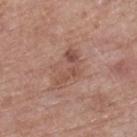Image and clinical context: A 15 mm crop from a total-body photograph taken for skin-cancer surveillance. A female patient, aged around 70. The lesion is on the right lower leg. Approximately 4.5 mm at its widest. Captured under white-light illumination.